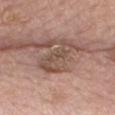This lesion was catalogued during total-body skin photography and was not selected for biopsy. A female patient, roughly 65 years of age. Automated tile analysis of the lesion measured an area of roughly 11 mm², an outline eccentricity of about 0.85 (0 = round, 1 = elongated), and a symmetry-axis asymmetry near 0.5. It also reported a lesion color around L≈50 a*≈17 b*≈25 in CIELAB and a lesion–skin lightness drop of about 9. The software also gave a border-irregularity index near 8/10 and a within-lesion color-variation index near 3.5/10. Captured under white-light illumination. A 15 mm close-up tile from a total-body photography series done for melanoma screening. On the chest. Measured at roughly 5.5 mm in maximum diameter.